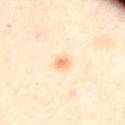Q: Is there a histopathology result?
A: no biopsy performed (imaged during a skin exam)
Q: Lesion location?
A: the upper back
Q: What is the imaging modality?
A: ~15 mm crop, total-body skin-cancer survey
Q: What are the patient's age and sex?
A: female, roughly 40 years of age
Q: Illumination type?
A: cross-polarized illumination
Q: What did automated image analysis measure?
A: a border-irregularity rating of about 2.5/10 and radial color variation of about 1